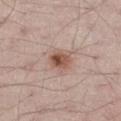Assessment:
Imaged during a routine full-body skin examination; the lesion was not biopsied and no histopathology is available.
Image and clinical context:
A male subject aged 38 to 42. A lesion tile, about 15 mm wide, cut from a 3D total-body photograph. The tile uses white-light illumination. The recorded lesion diameter is about 3.5 mm. The lesion is on the left thigh.